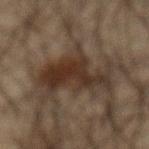Part of a total-body skin-imaging series; this lesion was reviewed on a skin check and was not flagged for biopsy.
The subject is a male aged approximately 65.
On the abdomen.
This image is a 15 mm lesion crop taken from a total-body photograph.
About 7 mm across.